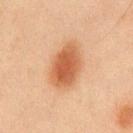Assessment:
Captured during whole-body skin photography for melanoma surveillance; the lesion was not biopsied.
Clinical summary:
The lesion is located on the chest. Imaged with cross-polarized lighting. The subject is a male about 45 years old. Automated tile analysis of the lesion measured an outline eccentricity of about 0.8 (0 = round, 1 = elongated) and a shape-asymmetry score of about 0.15 (0 = symmetric). The analysis additionally found a border-irregularity index near 2/10, a color-variation rating of about 3.5/10, and a peripheral color-asymmetry measure near 1. The software also gave a nevus-likeness score of about 100/100 and a lesion-detection confidence of about 100/100. A region of skin cropped from a whole-body photographic capture, roughly 15 mm wide.